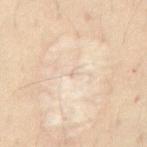Captured during whole-body skin photography for melanoma surveillance; the lesion was not biopsied.
A male subject about 45 years old.
Automated tile analysis of the lesion measured a lesion color around L≈74 a*≈12 b*≈27 in CIELAB and a normalized lesion–skin contrast near 3. It also reported a classifier nevus-likeness of about 0/100.
The lesion's longest dimension is about 1 mm.
The lesion is on the front of the torso.
Cropped from a total-body skin-imaging series; the visible field is about 15 mm.